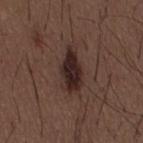biopsy status = catalogued during a skin exam; not biopsied | acquisition = total-body-photography crop, ~15 mm field of view | subject = male, aged around 50 | lighting = white-light illumination | image-analysis metrics = an average lesion color of about L≈25 a*≈16 b*≈18 (CIELAB), about 11 CIELAB-L* units darker than the surrounding skin, and a normalized lesion–skin contrast near 12; an automated nevus-likeness rating near 85 out of 100 and lesion-presence confidence of about 100/100 | location = the lower back | lesion size = ~5 mm (longest diameter).